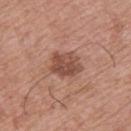{
  "biopsy_status": "not biopsied; imaged during a skin examination",
  "patient": {
    "sex": "male",
    "age_approx": 65
  },
  "automated_metrics": {
    "shape_asymmetry": 0.2
  },
  "site": "upper back",
  "image": {
    "source": "total-body photography crop",
    "field_of_view_mm": 15
  }
}